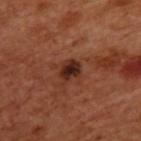Automated tile analysis of the lesion measured a lesion–skin lightness drop of about 12. The analysis additionally found border irregularity of about 2 on a 0–10 scale. The analysis additionally found an automated nevus-likeness rating near 70 out of 100 and lesion-presence confidence of about 100/100. Captured under cross-polarized illumination. A roughly 15 mm field-of-view crop from a total-body skin photograph. A male subject aged 48 to 52. Measured at roughly 2.5 mm in maximum diameter. From the upper back.